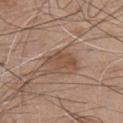Q: Automated lesion metrics?
A: an automated nevus-likeness rating near 5 out of 100 and lesion-presence confidence of about 100/100
Q: What is the lesion's diameter?
A: ~3.5 mm (longest diameter)
Q: Where on the body is the lesion?
A: the chest
Q: Patient demographics?
A: male, aged around 45
Q: What is the imaging modality?
A: ~15 mm crop, total-body skin-cancer survey